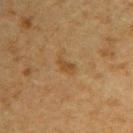workup = catalogued during a skin exam; not biopsied | site = the right upper arm | TBP lesion metrics = a shape eccentricity near 0.85; a mean CIELAB color near L≈38 a*≈15 b*≈32, about 6 CIELAB-L* units darker than the surrounding skin, and a normalized border contrast of about 6; an automated nevus-likeness rating near 5 out of 100 and lesion-presence confidence of about 100/100 | subject = male, aged approximately 50 | image = total-body-photography crop, ~15 mm field of view | lesion size = ~2.5 mm (longest diameter).